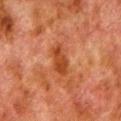  biopsy_status: not biopsied; imaged during a skin examination
  patient:
    sex: male
    age_approx: 80
  site: left lower leg
  image:
    source: total-body photography crop
    field_of_view_mm: 15
  lighting: cross-polarized